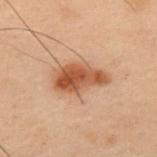* workup: catalogued during a skin exam; not biopsied
* illumination: cross-polarized illumination
* patient: male, roughly 55 years of age
* acquisition: 15 mm crop, total-body photography
* site: the upper back